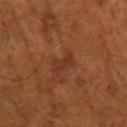{
  "biopsy_status": "not biopsied; imaged during a skin examination"
}